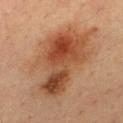Impression:
The lesion was tiled from a total-body skin photograph and was not biopsied.
Acquisition and patient details:
Longest diameter approximately 10 mm. A 15 mm crop from a total-body photograph taken for skin-cancer surveillance. From the upper back. The subject is a male aged approximately 35. Automated image analysis of the tile measured a mean CIELAB color near L≈45 a*≈22 b*≈32 and a normalized lesion–skin contrast near 8.5. It also reported a border-irregularity index near 4.5/10, internal color variation of about 9.5 on a 0–10 scale, and radial color variation of about 3.